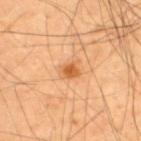{"image": {"source": "total-body photography crop", "field_of_view_mm": 15}, "patient": {"sex": "male", "age_approx": 55}, "site": "upper back", "lesion_size": {"long_diameter_mm_approx": 2.5}}